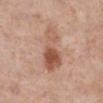No biopsy was performed on this lesion — it was imaged during a full skin examination and was not determined to be concerning. The subject is a female aged approximately 65. Located on the right lower leg. Captured under white-light illumination. This image is a 15 mm lesion crop taken from a total-body photograph. An algorithmic analysis of the crop reported an average lesion color of about L≈55 a*≈22 b*≈30 (CIELAB) and about 11 CIELAB-L* units darker than the surrounding skin. About 6 mm across.